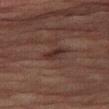Background:
Captured under cross-polarized illumination. A male subject about 85 years old or older. Cropped from a whole-body photographic skin survey; the tile spans about 15 mm. The lesion is located on the right thigh. The lesion's longest dimension is about 3 mm.